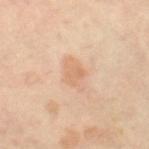The lesion was tiled from a total-body skin photograph and was not biopsied.
On the right thigh.
A female patient, aged 48–52.
The lesion's longest dimension is about 3.5 mm.
An algorithmic analysis of the crop reported an area of roughly 7 mm² and two-axis asymmetry of about 0.2. The analysis additionally found a mean CIELAB color near L≈70 a*≈20 b*≈34 and about 7 CIELAB-L* units darker than the surrounding skin. The software also gave peripheral color asymmetry of about 1. The software also gave a classifier nevus-likeness of about 0/100 and a lesion-detection confidence of about 100/100.
A close-up tile cropped from a whole-body skin photograph, about 15 mm across.